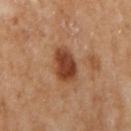Clinical impression:
Recorded during total-body skin imaging; not selected for excision or biopsy.
Background:
Automated image analysis of the tile measured a footprint of about 10 mm² and a shape-asymmetry score of about 0.15 (0 = symmetric). It also reported a lesion color around L≈42 a*≈24 b*≈33 in CIELAB, roughly 14 lightness units darker than nearby skin, and a normalized lesion–skin contrast near 10.5. It also reported a within-lesion color-variation index near 4/10 and radial color variation of about 1. The software also gave a detector confidence of about 100 out of 100 that the crop contains a lesion. A close-up tile cropped from a whole-body skin photograph, about 15 mm across. Imaged with cross-polarized lighting. Located on the right upper arm. A female subject, approximately 60 years of age.